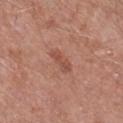No biopsy was performed on this lesion — it was imaged during a full skin examination and was not determined to be concerning. The subject is a male aged 58–62. The lesion is located on the left lower leg. Imaged with white-light lighting. Measured at roughly 3.5 mm in maximum diameter. A lesion tile, about 15 mm wide, cut from a 3D total-body photograph. The total-body-photography lesion software estimated an area of roughly 4 mm², an eccentricity of roughly 0.9, and a shape-asymmetry score of about 0.35 (0 = symmetric). The analysis additionally found a mean CIELAB color near L≈50 a*≈25 b*≈29, about 8 CIELAB-L* units darker than the surrounding skin, and a normalized border contrast of about 6.